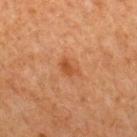Q: Was a biopsy performed?
A: total-body-photography surveillance lesion; no biopsy
Q: What lighting was used for the tile?
A: cross-polarized illumination
Q: Automated lesion metrics?
A: about 7 CIELAB-L* units darker than the surrounding skin and a lesion-to-skin contrast of about 6.5 (normalized; higher = more distinct); a border-irregularity index near 3/10, a color-variation rating of about 2.5/10, and radial color variation of about 1; a classifier nevus-likeness of about 20/100
Q: What is the imaging modality?
A: 15 mm crop, total-body photography
Q: What is the lesion's diameter?
A: ~2.5 mm (longest diameter)
Q: Lesion location?
A: the upper back
Q: What are the patient's age and sex?
A: male, about 65 years old